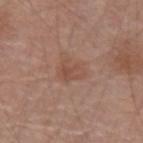Clinical impression: The lesion was tiled from a total-body skin photograph and was not biopsied.